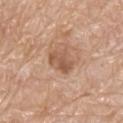A male subject, aged around 80.
The lesion-visualizer software estimated a lesion area of about 6 mm², an eccentricity of roughly 0.65, and two-axis asymmetry of about 0.3. And it measured a mean CIELAB color near L≈55 a*≈21 b*≈31, a lesion–skin lightness drop of about 10, and a lesion-to-skin contrast of about 7 (normalized; higher = more distinct). And it measured a border-irregularity rating of about 3.5/10, a color-variation rating of about 3/10, and peripheral color asymmetry of about 1.
On the back.
The recorded lesion diameter is about 3.5 mm.
A 15 mm close-up extracted from a 3D total-body photography capture.
This is a white-light tile.